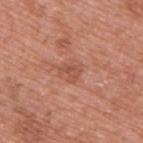Assessment: No biopsy was performed on this lesion — it was imaged during a full skin examination and was not determined to be concerning. Acquisition and patient details: The tile uses white-light illumination. About 2.5 mm across. The lesion is on the upper back. The patient is a male in their 70s. Automated image analysis of the tile measured a lesion area of about 4 mm², an eccentricity of roughly 0.65, and a symmetry-axis asymmetry near 0.45. The analysis additionally found a classifier nevus-likeness of about 0/100 and a detector confidence of about 100 out of 100 that the crop contains a lesion. A close-up tile cropped from a whole-body skin photograph, about 15 mm across.